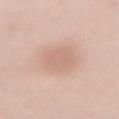Recorded during total-body skin imaging; not selected for excision or biopsy.
A 15 mm close-up extracted from a 3D total-body photography capture.
On the front of the torso.
The recorded lesion diameter is about 4.5 mm.
An algorithmic analysis of the crop reported about 7 CIELAB-L* units darker than the surrounding skin and a normalized lesion–skin contrast near 4.5.
The patient is a female in their mid- to late 40s.
This is a white-light tile.